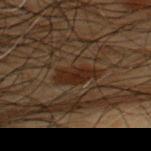Recorded during total-body skin imaging; not selected for excision or biopsy. A male subject approximately 50 years of age. The recorded lesion diameter is about 4.5 mm. From the upper back. A region of skin cropped from a whole-body photographic capture, roughly 15 mm wide.